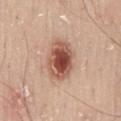<tbp_lesion>
<biopsy_status>not biopsied; imaged during a skin examination</biopsy_status>
<patient>
  <sex>male</sex>
  <age_approx>50</age_approx>
</patient>
<automated_metrics>
  <eccentricity>0.65</eccentricity>
  <border_irregularity_0_10>1.5</border_irregularity_0_10>
  <color_variation_0_10>8.5</color_variation_0_10>
  <peripheral_color_asymmetry>2.5</peripheral_color_asymmetry>
  <lesion_detection_confidence_0_100>100</lesion_detection_confidence_0_100>
</automated_metrics>
<lighting>white-light</lighting>
<image>
  <source>total-body photography crop</source>
  <field_of_view_mm>15</field_of_view_mm>
</image>
<site>mid back</site>
</tbp_lesion>